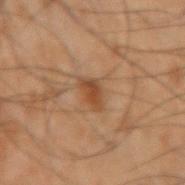| key | value |
|---|---|
| biopsy status | total-body-photography surveillance lesion; no biopsy |
| tile lighting | cross-polarized |
| image source | ~15 mm crop, total-body skin-cancer survey |
| location | the right upper arm |
| size | ~3 mm (longest diameter) |
| subject | male, aged approximately 50 |